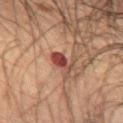notes: no biopsy performed (imaged during a skin exam).